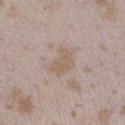Impression:
The lesion was photographed on a routine skin check and not biopsied; there is no pathology result.
Background:
On the left lower leg. Cropped from a whole-body photographic skin survey; the tile spans about 15 mm. The tile uses white-light illumination. The subject is a female roughly 25 years of age. About 3.5 mm across.